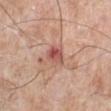location — the left lower leg | lighting — white-light | image — ~15 mm crop, total-body skin-cancer survey | automated lesion analysis — a lesion area of about 4.5 mm² and a shape eccentricity near 0.7; a border-irregularity rating of about 3/10 | patient — male, aged 73 to 77.